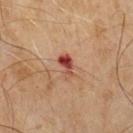{
  "biopsy_status": "not biopsied; imaged during a skin examination",
  "lesion_size": {
    "long_diameter_mm_approx": 3.0
  },
  "patient": {
    "sex": "male",
    "age_approx": 65
  },
  "lighting": "cross-polarized",
  "image": {
    "source": "total-body photography crop",
    "field_of_view_mm": 15
  }
}